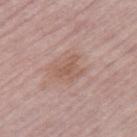Findings:
• lighting — white-light
• location — the left leg
• subject — female, aged 63–67
• image — 15 mm crop, total-body photography